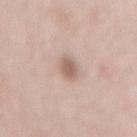Assessment: No biopsy was performed on this lesion — it was imaged during a full skin examination and was not determined to be concerning. Background: A female patient roughly 70 years of age. The lesion is located on the mid back. A close-up tile cropped from a whole-body skin photograph, about 15 mm across. Captured under white-light illumination. An algorithmic analysis of the crop reported an area of roughly 4 mm² and two-axis asymmetry of about 0.3.